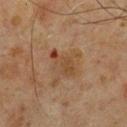Recorded during total-body skin imaging; not selected for excision or biopsy. The tile uses cross-polarized illumination. The lesion is located on the chest. A 15 mm close-up tile from a total-body photography series done for melanoma screening. A male subject, aged 58 to 62.